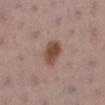Captured during whole-body skin photography for melanoma surveillance; the lesion was not biopsied. This is a white-light tile. The patient is a female about 55 years old. Located on the left lower leg. Approximately 3.5 mm at its widest. A roughly 15 mm field-of-view crop from a total-body skin photograph.